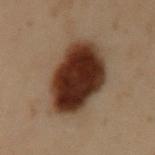This lesion was catalogued during total-body skin photography and was not selected for biopsy. Captured under cross-polarized illumination. A region of skin cropped from a whole-body photographic capture, roughly 15 mm wide. The lesion's longest dimension is about 6.5 mm. Located on the left upper arm. The patient is a male approximately 55 years of age.